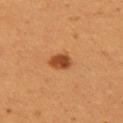| field | value |
|---|---|
| biopsy status | imaged on a skin check; not biopsied |
| tile lighting | cross-polarized illumination |
| lesion diameter | ~3 mm (longest diameter) |
| anatomic site | the arm |
| imaging modality | 15 mm crop, total-body photography |
| patient | female, aged around 25 |
| image-analysis metrics | a lesion–skin lightness drop of about 13 and a lesion-to-skin contrast of about 9.5 (normalized; higher = more distinct); a border-irregularity rating of about 2.5/10, a within-lesion color-variation index near 3/10, and radial color variation of about 1 |